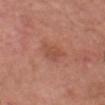Imaged during a routine full-body skin examination; the lesion was not biopsied and no histopathology is available.
A male patient, about 60 years old.
Located on the head or neck.
This is a white-light tile.
Cropped from a whole-body photographic skin survey; the tile spans about 15 mm.
About 3 mm across.